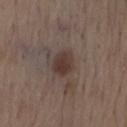- illumination · white-light illumination
- imaging modality · total-body-photography crop, ~15 mm field of view
- patient · male, about 70 years old
- site · the mid back